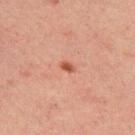– follow-up: total-body-photography surveillance lesion; no biopsy
– lesion diameter: ~1.5 mm (longest diameter)
– image-analysis metrics: an outline eccentricity of about 0.75 (0 = round, 1 = elongated) and a shape-asymmetry score of about 0.2 (0 = symmetric); about 10 CIELAB-L* units darker than the surrounding skin and a lesion-to-skin contrast of about 8.5 (normalized; higher = more distinct); a border-irregularity rating of about 1.5/10 and a color-variation rating of about 0/10; a classifier nevus-likeness of about 85/100
– location: the upper back
– subject: male, in their 30s
– image: total-body-photography crop, ~15 mm field of view